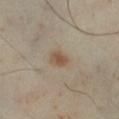follow-up = no biopsy performed (imaged during a skin exam); illumination = cross-polarized illumination; lesion size = about 2.5 mm; body site = the right lower leg; patient = male, approximately 40 years of age; imaging modality = 15 mm crop, total-body photography.